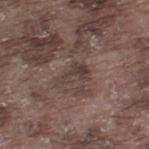Automated tile analysis of the lesion measured an area of roughly 5 mm², an outline eccentricity of about 0.9 (0 = round, 1 = elongated), and two-axis asymmetry of about 0.6. It also reported an average lesion color of about L≈39 a*≈15 b*≈19 (CIELAB), a lesion–skin lightness drop of about 7, and a normalized border contrast of about 6.5. The analysis additionally found a nevus-likeness score of about 0/100 and a lesion-detection confidence of about 70/100. The subject is a male aged 73 to 77. The recorded lesion diameter is about 4 mm. Captured under white-light illumination. On the left thigh. Cropped from a whole-body photographic skin survey; the tile spans about 15 mm.